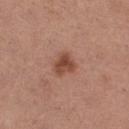Q: Is there a histopathology result?
A: imaged on a skin check; not biopsied
Q: What is the imaging modality?
A: ~15 mm tile from a whole-body skin photo
Q: What is the anatomic site?
A: the right thigh
Q: What lighting was used for the tile?
A: white-light illumination
Q: Patient demographics?
A: female, aged around 30
Q: Lesion size?
A: about 3 mm
Q: What did automated image analysis measure?
A: a lesion area of about 5.5 mm² and a symmetry-axis asymmetry near 0.35; a mean CIELAB color near L≈47 a*≈23 b*≈29, roughly 11 lightness units darker than nearby skin, and a normalized border contrast of about 8.5